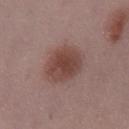location = the right thigh | lighting = white-light | imaging modality = 15 mm crop, total-body photography | image-analysis metrics = a lesion area of about 15 mm² and two-axis asymmetry of about 0.15; an automated nevus-likeness rating near 100 out of 100 and lesion-presence confidence of about 100/100 | lesion diameter = about 5 mm | patient = female, aged 28 to 32.